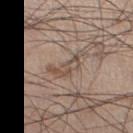| key | value |
|---|---|
| location | the left thigh |
| acquisition | ~15 mm crop, total-body skin-cancer survey |
| subject | male, in their mid- to late 50s |